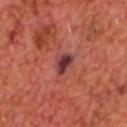Assessment: No biopsy was performed on this lesion — it was imaged during a full skin examination and was not determined to be concerning. Acquisition and patient details: About 3 mm across. A male patient, aged 63–67. Cropped from a whole-body photographic skin survey; the tile spans about 15 mm. Imaged with cross-polarized lighting.